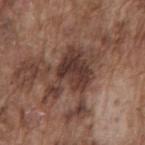Captured during whole-body skin photography for melanoma surveillance; the lesion was not biopsied.
Cropped from a whole-body photographic skin survey; the tile spans about 15 mm.
Imaged with white-light lighting.
A male patient, approximately 75 years of age.
Located on the mid back.